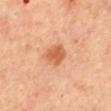follow-up — total-body-photography surveillance lesion; no biopsy | image — total-body-photography crop, ~15 mm field of view | anatomic site — the mid back | lesion size — about 3.5 mm | patient — female, in their mid- to late 40s | TBP lesion metrics — an area of roughly 6.5 mm² and an outline eccentricity of about 0.7 (0 = round, 1 = elongated); a border-irregularity rating of about 2.5/10 and peripheral color asymmetry of about 0.5.